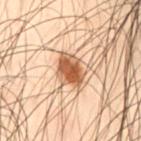Assessment: Captured during whole-body skin photography for melanoma surveillance; the lesion was not biopsied. Acquisition and patient details: Automated image analysis of the tile measured an area of roughly 8.5 mm² and an outline eccentricity of about 0.5 (0 = round, 1 = elongated). It also reported a mean CIELAB color near L≈44 a*≈19 b*≈29. And it measured a nevus-likeness score of about 100/100 and a lesion-detection confidence of about 100/100. From the right thigh. Imaged with cross-polarized lighting. A 15 mm close-up tile from a total-body photography series done for melanoma screening. The lesion's longest dimension is about 3.5 mm. A male subject in their 50s.